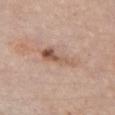Findings:
• biopsy status · catalogued during a skin exam; not biopsied
• lesion size · ~5.5 mm (longest diameter)
• automated metrics · an outline eccentricity of about 0.9 (0 = round, 1 = elongated) and a shape-asymmetry score of about 0.35 (0 = symmetric); a nevus-likeness score of about 70/100 and a detector confidence of about 100 out of 100 that the crop contains a lesion
• patient · female, in their mid- to late 60s
• image · 15 mm crop, total-body photography
• body site · the chest